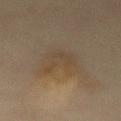Captured during whole-body skin photography for melanoma surveillance; the lesion was not biopsied.